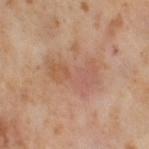workup: catalogued during a skin exam; not biopsied
tile lighting: cross-polarized illumination
patient: female, aged approximately 55
automated metrics: a lesion area of about 12 mm², an eccentricity of roughly 0.85, and a shape-asymmetry score of about 0.5 (0 = symmetric); a border-irregularity rating of about 7/10 and internal color variation of about 5.5 on a 0–10 scale
site: the right thigh
image: total-body-photography crop, ~15 mm field of view